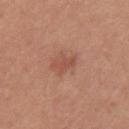biopsy status: no biopsy performed (imaged during a skin exam) | TBP lesion metrics: an area of roughly 3.5 mm², an outline eccentricity of about 0.75 (0 = round, 1 = elongated), and a shape-asymmetry score of about 0.35 (0 = symmetric); a lesion color around L≈51 a*≈25 b*≈30 in CIELAB and a normalized lesion–skin contrast near 5.5; a within-lesion color-variation index near 1.5/10 and radial color variation of about 0.5; an automated nevus-likeness rating near 20 out of 100 | lighting: white-light illumination | image source: total-body-photography crop, ~15 mm field of view | subject: female, aged 28–32 | size: ≈2.5 mm | site: the left upper arm.